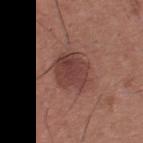Assessment:
The lesion was tiled from a total-body skin photograph and was not biopsied.
Acquisition and patient details:
Cropped from a whole-body photographic skin survey; the tile spans about 15 mm. On the upper back. A male patient, roughly 35 years of age.